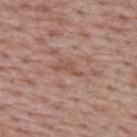Impression:
Recorded during total-body skin imaging; not selected for excision or biopsy.
Acquisition and patient details:
From the upper back. A 15 mm close-up extracted from a 3D total-body photography capture. A male patient, in their mid-60s. An algorithmic analysis of the crop reported an average lesion color of about L≈52 a*≈21 b*≈27 (CIELAB). The software also gave border irregularity of about 8 on a 0–10 scale, a color-variation rating of about 0/10, and a peripheral color-asymmetry measure near 0.